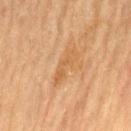{
  "biopsy_status": "not biopsied; imaged during a skin examination",
  "image": {
    "source": "total-body photography crop",
    "field_of_view_mm": 15
  },
  "site": "lower back",
  "lighting": "cross-polarized",
  "lesion_size": {
    "long_diameter_mm_approx": 4.5
  },
  "patient": {
    "sex": "male",
    "age_approx": "85+"
  },
  "automated_metrics": {
    "eccentricity": 0.95,
    "shape_asymmetry": 0.4,
    "cielab_L": 52,
    "cielab_a": 19,
    "cielab_b": 36,
    "vs_skin_darker_L": 6.0,
    "vs_skin_contrast_norm": 5.0
  }
}